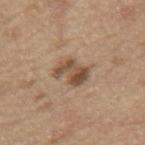Q: Is there a histopathology result?
A: imaged on a skin check; not biopsied
Q: What is the imaging modality?
A: ~15 mm crop, total-body skin-cancer survey
Q: Lesion size?
A: ~3.5 mm (longest diameter)
Q: What lighting was used for the tile?
A: white-light
Q: Where on the body is the lesion?
A: the back
Q: What are the patient's age and sex?
A: male, aged 68–72